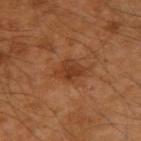{
  "biopsy_status": "not biopsied; imaged during a skin examination",
  "automated_metrics": {
    "area_mm2_approx": 6.0,
    "shape_asymmetry": 0.25
  },
  "lesion_size": {
    "long_diameter_mm_approx": 3.0
  },
  "patient": {
    "sex": "male",
    "age_approx": 50
  },
  "image": {
    "source": "total-body photography crop",
    "field_of_view_mm": 15
  },
  "site": "arm"
}